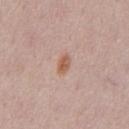Clinical impression: No biopsy was performed on this lesion — it was imaged during a full skin examination and was not determined to be concerning. Background: From the chest. The total-body-photography lesion software estimated a footprint of about 3.5 mm², an eccentricity of roughly 0.8, and a shape-asymmetry score of about 0.15 (0 = symmetric). The analysis additionally found a mean CIELAB color near L≈58 a*≈21 b*≈30, roughly 9 lightness units darker than nearby skin, and a normalized border contrast of about 7.5. It also reported a detector confidence of about 100 out of 100 that the crop contains a lesion. A male subject, aged 48–52. The lesion's longest dimension is about 2.5 mm. Cropped from a whole-body photographic skin survey; the tile spans about 15 mm.